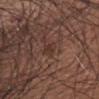follow-up: total-body-photography surveillance lesion; no biopsy